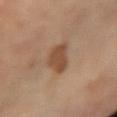The lesion was photographed on a routine skin check and not biopsied; there is no pathology result.
A 15 mm crop from a total-body photograph taken for skin-cancer surveillance.
A female patient approximately 65 years of age.
The lesion is located on the left arm.